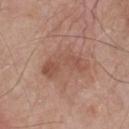The lesion was tiled from a total-body skin photograph and was not biopsied. A male patient, roughly 80 years of age. Automated tile analysis of the lesion measured a mean CIELAB color near L≈52 a*≈21 b*≈28, roughly 8 lightness units darker than nearby skin, and a normalized border contrast of about 5.5. The software also gave a border-irregularity index near 5.5/10, a color-variation rating of about 3.5/10, and radial color variation of about 1. It also reported a nevus-likeness score of about 0/100. This image is a 15 mm lesion crop taken from a total-body photograph. Imaged with white-light lighting. The lesion is on the chest. About 6 mm across.